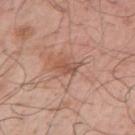follow-up: no biopsy performed (imaged during a skin exam)
anatomic site: the right upper arm
TBP lesion metrics: a footprint of about 3.5 mm² and an outline eccentricity of about 0.8 (0 = round, 1 = elongated); a lesion–skin lightness drop of about 8; a nevus-likeness score of about 5/100 and a lesion-detection confidence of about 100/100
lesion size: ~2.5 mm (longest diameter)
image: ~15 mm tile from a whole-body skin photo
tile lighting: white-light
subject: male, aged 38 to 42